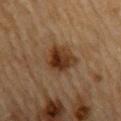Q: Was a biopsy performed?
A: no biopsy performed (imaged during a skin exam)
Q: What did automated image analysis measure?
A: a lesion color around L≈30 a*≈16 b*≈27 in CIELAB and about 10 CIELAB-L* units darker than the surrounding skin
Q: How was the tile lit?
A: cross-polarized illumination
Q: What are the patient's age and sex?
A: male, aged 83 or over
Q: What is the imaging modality?
A: ~15 mm crop, total-body skin-cancer survey
Q: How large is the lesion?
A: ≈4 mm
Q: Where on the body is the lesion?
A: the mid back